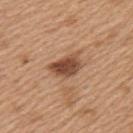Assessment: This lesion was catalogued during total-body skin photography and was not selected for biopsy. Clinical summary: From the left upper arm. Captured under white-light illumination. Cropped from a whole-body photographic skin survey; the tile spans about 15 mm. The patient is a female roughly 40 years of age.